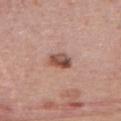Approximately 3 mm at its widest.
A male patient, about 40 years old.
Imaged with white-light lighting.
On the upper back.
An algorithmic analysis of the crop reported a footprint of about 5.5 mm². It also reported an average lesion color of about L≈51 a*≈22 b*≈27 (CIELAB), about 14 CIELAB-L* units darker than the surrounding skin, and a normalized lesion–skin contrast near 9.5. The analysis additionally found a border-irregularity index near 1.5/10 and internal color variation of about 7.5 on a 0–10 scale. It also reported an automated nevus-likeness rating near 90 out of 100.
A 15 mm close-up extracted from a 3D total-body photography capture.